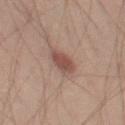biopsy status: total-body-photography surveillance lesion; no biopsy | anatomic site: the left thigh | subject: male, roughly 40 years of age | lesion size: ~3 mm (longest diameter) | imaging modality: ~15 mm tile from a whole-body skin photo | automated lesion analysis: an average lesion color of about L≈37 a*≈17 b*≈20 (CIELAB) and a lesion-to-skin contrast of about 8 (normalized; higher = more distinct); a border-irregularity rating of about 2.5/10 and a color-variation rating of about 2.5/10.